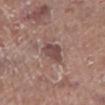Case summary:
– lesion diameter — about 3 mm
– image source — 15 mm crop, total-body photography
– anatomic site — the left lower leg
– lighting — white-light
– subject — male, roughly 70 years of age